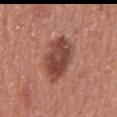follow-up = total-body-photography surveillance lesion; no biopsy | anatomic site = the mid back | subject = male, roughly 65 years of age | image = 15 mm crop, total-body photography.